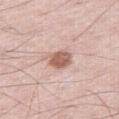Part of a total-body skin-imaging series; this lesion was reviewed on a skin check and was not flagged for biopsy. A 15 mm close-up extracted from a 3D total-body photography capture. A male subject, about 60 years old. The lesion is on the right thigh. Longest diameter approximately 3 mm. Automated image analysis of the tile measured a lesion–skin lightness drop of about 14 and a normalized lesion–skin contrast near 9. The analysis additionally found a border-irregularity index near 2/10 and internal color variation of about 3 on a 0–10 scale. And it measured an automated nevus-likeness rating near 85 out of 100 and a detector confidence of about 100 out of 100 that the crop contains a lesion.